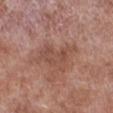Recorded during total-body skin imaging; not selected for excision or biopsy.
About 5 mm across.
Automated tile analysis of the lesion measured a footprint of about 10 mm², an outline eccentricity of about 0.8 (0 = round, 1 = elongated), and a shape-asymmetry score of about 0.45 (0 = symmetric). And it measured a mean CIELAB color near L≈49 a*≈22 b*≈27 and a lesion–skin lightness drop of about 7. The analysis additionally found a classifier nevus-likeness of about 0/100 and lesion-presence confidence of about 100/100.
This is a white-light tile.
On the right lower leg.
The subject is a male aged 53 to 57.
A 15 mm close-up extracted from a 3D total-body photography capture.